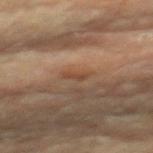Notes:
– notes: no biopsy performed (imaged during a skin exam)
– image: total-body-photography crop, ~15 mm field of view
– location: the back
– illumination: cross-polarized illumination
– subject: female, in their 80s
– automated metrics: an outline eccentricity of about 0.95 (0 = round, 1 = elongated) and a symmetry-axis asymmetry near 0.35; a lesion color around L≈41 a*≈18 b*≈28 in CIELAB and a normalized border contrast of about 6; a nevus-likeness score of about 0/100 and a lesion-detection confidence of about 85/100